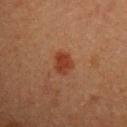Findings:
• follow-up — catalogued during a skin exam; not biopsied
• illumination — cross-polarized illumination
• patient — male, aged approximately 40
• lesion diameter — ≈3 mm
• location — the chest
• automated metrics — a lesion color around L≈32 a*≈24 b*≈28 in CIELAB, roughly 8 lightness units darker than nearby skin, and a lesion-to-skin contrast of about 8 (normalized; higher = more distinct); lesion-presence confidence of about 100/100
• image source — total-body-photography crop, ~15 mm field of view